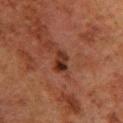notes = catalogued during a skin exam; not biopsied | anatomic site = the leg | image = 15 mm crop, total-body photography | TBP lesion metrics = an area of roughly 4 mm², an eccentricity of roughly 0.8, and two-axis asymmetry of about 0.3; an average lesion color of about L≈24 a*≈19 b*≈23 (CIELAB), a lesion–skin lightness drop of about 9, and a normalized border contrast of about 10; border irregularity of about 3 on a 0–10 scale and a within-lesion color-variation index near 7.5/10 | subject = male, in their 80s | illumination = cross-polarized.